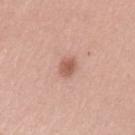| key | value |
|---|---|
| patient | female, aged 33–37 |
| body site | the left upper arm |
| automated metrics | a lesion area of about 4 mm², an eccentricity of roughly 0.7, and a symmetry-axis asymmetry near 0.25; a mean CIELAB color near L≈58 a*≈23 b*≈28, a lesion–skin lightness drop of about 11, and a normalized border contrast of about 7.5; a nevus-likeness score of about 80/100 and lesion-presence confidence of about 100/100 |
| lesion size | about 2.5 mm |
| illumination | white-light |
| acquisition | 15 mm crop, total-body photography |